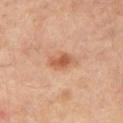Captured during whole-body skin photography for melanoma surveillance; the lesion was not biopsied. On the right thigh. The tile uses cross-polarized illumination. Measured at roughly 3.5 mm in maximum diameter. Cropped from a total-body skin-imaging series; the visible field is about 15 mm. A male subject in their mid-50s.